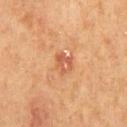Findings:
- biopsy status: catalogued during a skin exam; not biopsied
- subject: male, approximately 65 years of age
- acquisition: 15 mm crop, total-body photography
- lesion size: about 2.5 mm
- body site: the back
- tile lighting: cross-polarized illumination
- automated lesion analysis: a shape eccentricity near 0.55 and a shape-asymmetry score of about 0.25 (0 = symmetric); an average lesion color of about L≈50 a*≈24 b*≈33 (CIELAB) and a normalized lesion–skin contrast near 5.5; border irregularity of about 2.5 on a 0–10 scale, internal color variation of about 5 on a 0–10 scale, and radial color variation of about 1.5; an automated nevus-likeness rating near 0 out of 100 and a detector confidence of about 100 out of 100 that the crop contains a lesion